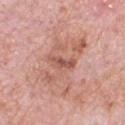{"biopsy_status": "not biopsied; imaged during a skin examination", "image": {"source": "total-body photography crop", "field_of_view_mm": 15}, "site": "chest", "automated_metrics": {"color_variation_0_10": 3.0}, "lighting": "white-light", "patient": {"sex": "male", "age_approx": 80}, "lesion_size": {"long_diameter_mm_approx": 3.0}}